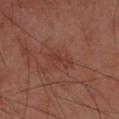Clinical impression:
Part of a total-body skin-imaging series; this lesion was reviewed on a skin check and was not flagged for biopsy.